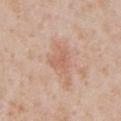The lesion was tiled from a total-body skin photograph and was not biopsied.
A 15 mm close-up extracted from a 3D total-body photography capture.
Located on the front of the torso.
A male subject, in their mid- to late 60s.
The lesion's longest dimension is about 2.5 mm.
The tile uses white-light illumination.